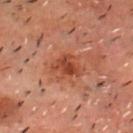Case summary:
* follow-up · catalogued during a skin exam; not biopsied
* illumination · cross-polarized
* lesion diameter · ~3.5 mm (longest diameter)
* image source · ~15 mm crop, total-body skin-cancer survey
* location · the head or neck
* subject · male, about 50 years old
* automated lesion analysis · a lesion color around L≈34 a*≈23 b*≈27 in CIELAB, about 8 CIELAB-L* units darker than the surrounding skin, and a normalized border contrast of about 7.5; a color-variation rating of about 3.5/10 and peripheral color asymmetry of about 1